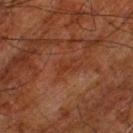Findings:
• biopsy status — no biopsy performed (imaged during a skin exam)
• subject — male, aged 78 to 82
• imaging modality — total-body-photography crop, ~15 mm field of view
• site — the left thigh